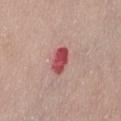Captured during whole-body skin photography for melanoma surveillance; the lesion was not biopsied. On the abdomen. This is a white-light tile. Approximately 3.5 mm at its widest. A 15 mm close-up extracted from a 3D total-body photography capture. A female subject in their 80s.